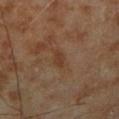{
  "biopsy_status": "not biopsied; imaged during a skin examination",
  "image": {
    "source": "total-body photography crop",
    "field_of_view_mm": 15
  },
  "lighting": "cross-polarized",
  "site": "right lower leg",
  "lesion_size": {
    "long_diameter_mm_approx": 2.5
  },
  "patient": {
    "sex": "male",
    "age_approx": 70
  }
}